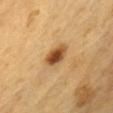The lesion was tiled from a total-body skin photograph and was not biopsied.
On the chest.
A 15 mm close-up extracted from a 3D total-body photography capture.
The patient is a male aged 58 to 62.